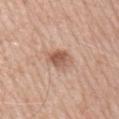Captured during whole-body skin photography for melanoma surveillance; the lesion was not biopsied. Located on the arm. Approximately 2.5 mm at its widest. A male patient, in their 60s. A 15 mm close-up tile from a total-body photography series done for melanoma screening. This is a white-light tile. The total-body-photography lesion software estimated a lesion area of about 5.5 mm², an outline eccentricity of about 0.45 (0 = round, 1 = elongated), and a symmetry-axis asymmetry near 0.2. The software also gave an average lesion color of about L≈56 a*≈21 b*≈29 (CIELAB), roughly 12 lightness units darker than nearby skin, and a normalized lesion–skin contrast near 8.